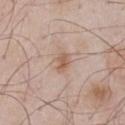Notes:
- biopsy status: imaged on a skin check; not biopsied
- site: the chest
- image: ~15 mm tile from a whole-body skin photo
- illumination: white-light illumination
- size: ~2.5 mm (longest diameter)
- automated metrics: an average lesion color of about L≈58 a*≈18 b*≈28 (CIELAB), a lesion–skin lightness drop of about 9, and a normalized border contrast of about 7
- patient: male, aged 53 to 57